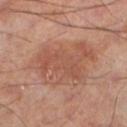Q: What kind of image is this?
A: 15 mm crop, total-body photography
Q: What are the patient's age and sex?
A: male, in their mid- to late 50s
Q: What lighting was used for the tile?
A: cross-polarized illumination
Q: Where on the body is the lesion?
A: the left lower leg
Q: What is the lesion's diameter?
A: about 8.5 mm